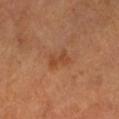Recorded during total-body skin imaging; not selected for excision or biopsy. On the left lower leg. A male subject, aged around 55. A 15 mm close-up tile from a total-body photography series done for melanoma screening. About 3 mm across. This is a cross-polarized tile.